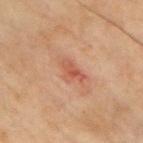{
  "biopsy_status": "not biopsied; imaged during a skin examination",
  "patient": {
    "sex": "male",
    "age_approx": 55
  },
  "automated_metrics": {
    "area_mm2_approx": 4.5,
    "eccentricity": 0.8,
    "cielab_L": 44,
    "cielab_a": 23,
    "cielab_b": 27,
    "vs_skin_darker_L": 8.0,
    "vs_skin_contrast_norm": 6.0,
    "peripheral_color_asymmetry": 0.5,
    "nevus_likeness_0_100": 0,
    "lesion_detection_confidence_0_100": 100
  },
  "image": {
    "source": "total-body photography crop",
    "field_of_view_mm": 15
  },
  "lesion_size": {
    "long_diameter_mm_approx": 3.5
  },
  "lighting": "cross-polarized",
  "site": "chest"
}